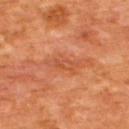Clinical summary:
A roughly 15 mm field-of-view crop from a total-body skin photograph. Longest diameter approximately 3.5 mm. A male subject in their mid- to late 60s. The lesion is on the back. Imaged with cross-polarized lighting. An algorithmic analysis of the crop reported an area of roughly 4 mm², an eccentricity of roughly 0.9, and a shape-asymmetry score of about 0.4 (0 = symmetric). And it measured an average lesion color of about L≈51 a*≈29 b*≈37 (CIELAB), a lesion–skin lightness drop of about 7, and a normalized border contrast of about 5. It also reported a border-irregularity index near 4.5/10, internal color variation of about 3 on a 0–10 scale, and radial color variation of about 1.